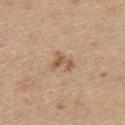No biopsy was performed on this lesion — it was imaged during a full skin examination and was not determined to be concerning. A 15 mm close-up tile from a total-body photography series done for melanoma screening. On the upper back. A female patient, roughly 45 years of age.